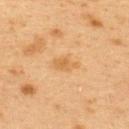<tbp_lesion>
  <biopsy_status>not biopsied; imaged during a skin examination</biopsy_status>
  <patient>
    <sex>female</sex>
    <age_approx>40</age_approx>
  </patient>
  <site>upper back</site>
  <image>
    <source>total-body photography crop</source>
    <field_of_view_mm>15</field_of_view_mm>
  </image>
</tbp_lesion>